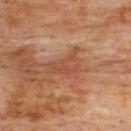biopsy status=catalogued during a skin exam; not biopsied
patient=male, aged 73–77
lesion diameter=about 5 mm
acquisition=~15 mm tile from a whole-body skin photo
body site=the upper back